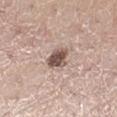follow-up — no biopsy performed (imaged during a skin exam)